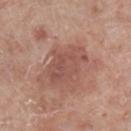The lesion was photographed on a routine skin check and not biopsied; there is no pathology result. A 15 mm close-up extracted from a 3D total-body photography capture. Longest diameter approximately 5.5 mm. A male subject, aged approximately 70. On the left lower leg. Imaged with white-light lighting.